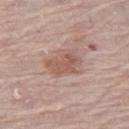{
  "biopsy_status": "not biopsied; imaged during a skin examination",
  "automated_metrics": {
    "eccentricity": 0.8,
    "cielab_L": 55,
    "cielab_a": 20,
    "cielab_b": 25,
    "vs_skin_contrast_norm": 6.5,
    "border_irregularity_0_10": 3.5,
    "color_variation_0_10": 3.0,
    "peripheral_color_asymmetry": 1.0
  },
  "lesion_size": {
    "long_diameter_mm_approx": 4.0
  },
  "image": {
    "source": "total-body photography crop",
    "field_of_view_mm": 15
  },
  "site": "left thigh",
  "patient": {
    "sex": "female",
    "age_approx": 65
  },
  "lighting": "white-light"
}